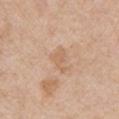follow-up — no biopsy performed (imaged during a skin exam) | patient — male, roughly 65 years of age | lesion size — ~3 mm (longest diameter) | image — total-body-photography crop, ~15 mm field of view | location — the left upper arm.